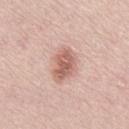Clinical impression: Part of a total-body skin-imaging series; this lesion was reviewed on a skin check and was not flagged for biopsy. Image and clinical context: Imaged with white-light lighting. A close-up tile cropped from a whole-body skin photograph, about 15 mm across. The lesion is located on the lower back. A female patient in their mid-60s.